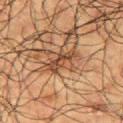Clinical impression:
The lesion was photographed on a routine skin check and not biopsied; there is no pathology result.
Clinical summary:
Approximately 4 mm at its widest. A region of skin cropped from a whole-body photographic capture, roughly 15 mm wide. The lesion is on the right upper arm. The patient is a male in their 60s. Captured under cross-polarized illumination.